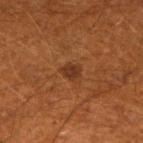Q: Was this lesion biopsied?
A: imaged on a skin check; not biopsied
Q: How was this image acquired?
A: ~15 mm tile from a whole-body skin photo
Q: What is the anatomic site?
A: the left lower leg
Q: Patient demographics?
A: male, in their 60s
Q: Illumination type?
A: cross-polarized illumination
Q: What is the lesion's diameter?
A: ~2.5 mm (longest diameter)